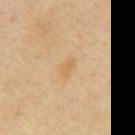Recorded during total-body skin imaging; not selected for excision or biopsy.